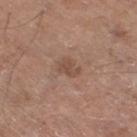Part of a total-body skin-imaging series; this lesion was reviewed on a skin check and was not flagged for biopsy. This is a white-light tile. Cropped from a whole-body photographic skin survey; the tile spans about 15 mm. Located on the right thigh. Automated image analysis of the tile measured a mean CIELAB color near L≈49 a*≈19 b*≈27, roughly 8 lightness units darker than nearby skin, and a lesion-to-skin contrast of about 6 (normalized; higher = more distinct). It also reported a border-irregularity index near 3/10 and a within-lesion color-variation index near 1/10. The subject is a male about 65 years old.